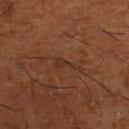Assessment: Recorded during total-body skin imaging; not selected for excision or biopsy. Image and clinical context: On the right lower leg. A 15 mm close-up extracted from a 3D total-body photography capture. The patient is a male aged around 65.